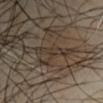The tile uses cross-polarized illumination. Measured at roughly 4 mm in maximum diameter. The lesion is on the right upper arm. The subject is a male in their 40s. A close-up tile cropped from a whole-body skin photograph, about 15 mm across.